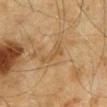<lesion>
  <biopsy_status>not biopsied; imaged during a skin examination</biopsy_status>
  <lighting>cross-polarized</lighting>
  <site>mid back</site>
  <image>
    <source>total-body photography crop</source>
    <field_of_view_mm>15</field_of_view_mm>
  </image>
  <patient>
    <sex>male</sex>
    <age_approx>65</age_approx>
  </patient>
  <lesion_size>
    <long_diameter_mm_approx>3.0</long_diameter_mm_approx>
  </lesion_size>
</lesion>